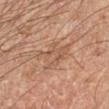<lesion>
<biopsy_status>not biopsied; imaged during a skin examination</biopsy_status>
<lesion_size>
  <long_diameter_mm_approx>5.0</long_diameter_mm_approx>
</lesion_size>
<site>left forearm</site>
<image>
  <source>total-body photography crop</source>
  <field_of_view_mm>15</field_of_view_mm>
</image>
<lighting>cross-polarized</lighting>
<patient>
  <sex>male</sex>
  <age_approx>60</age_approx>
</patient>
<automated_metrics>
  <shape_asymmetry>0.45</shape_asymmetry>
  <cielab_L>52</cielab_L>
  <cielab_a>20</cielab_a>
  <cielab_b>31</cielab_b>
  <vs_skin_darker_L>7.0</vs_skin_darker_L>
  <vs_skin_contrast_norm>5.0</vs_skin_contrast_norm>
  <border_irregularity_0_10>6.0</border_irregularity_0_10>
  <color_variation_0_10>3.0</color_variation_0_10>
  <peripheral_color_asymmetry>1.0</peripheral_color_asymmetry>
  <lesion_detection_confidence_0_100>70</lesion_detection_confidence_0_100>
</automated_metrics>
</lesion>